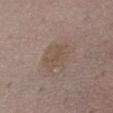* notes · imaged on a skin check; not biopsied
* patient · female, aged 48–52
* image · 15 mm crop, total-body photography
* automated lesion analysis · a lesion area of about 9 mm², an eccentricity of roughly 0.65, and a symmetry-axis asymmetry near 0.2; an average lesion color of about L≈50 a*≈14 b*≈25 (CIELAB), about 6 CIELAB-L* units darker than the surrounding skin, and a normalized lesion–skin contrast near 5.5
* lighting · white-light illumination
* body site · the chest